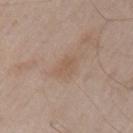Q: Was a biopsy performed?
A: catalogued during a skin exam; not biopsied
Q: Who is the patient?
A: male, about 55 years old
Q: Where on the body is the lesion?
A: the arm
Q: What is the imaging modality?
A: 15 mm crop, total-body photography